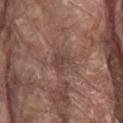Clinical impression: Part of a total-body skin-imaging series; this lesion was reviewed on a skin check and was not flagged for biopsy. Acquisition and patient details: A 15 mm crop from a total-body photograph taken for skin-cancer surveillance. A male subject aged 78 to 82. The lesion is on the back.